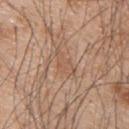Assessment: The lesion was photographed on a routine skin check and not biopsied; there is no pathology result. Context: Automated tile analysis of the lesion measured about 6 CIELAB-L* units darker than the surrounding skin and a normalized lesion–skin contrast near 4.5. It also reported internal color variation of about 1 on a 0–10 scale and radial color variation of about 0.5. The software also gave an automated nevus-likeness rating near 0 out of 100 and lesion-presence confidence of about 50/100. The lesion is located on the upper back. A male patient, roughly 50 years of age. A region of skin cropped from a whole-body photographic capture, roughly 15 mm wide. The recorded lesion diameter is about 4 mm.